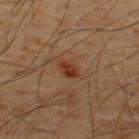notes — no biopsy performed (imaged during a skin exam)
tile lighting — cross-polarized
acquisition — ~15 mm tile from a whole-body skin photo
anatomic site — the upper back
subject — male, aged 53–57
size — about 2.5 mm